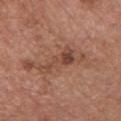follow-up: catalogued during a skin exam; not biopsied
subject: male, in their mid- to late 70s
location: the chest
lighting: white-light illumination
diameter: about 5 mm
acquisition: 15 mm crop, total-body photography
TBP lesion metrics: an average lesion color of about L≈45 a*≈22 b*≈28 (CIELAB) and a lesion–skin lightness drop of about 9; a border-irregularity rating of about 8/10 and a color-variation rating of about 3.5/10; an automated nevus-likeness rating near 5 out of 100 and a lesion-detection confidence of about 100/100